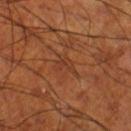The lesion was photographed on a routine skin check and not biopsied; there is no pathology result. Located on the right thigh. A region of skin cropped from a whole-body photographic capture, roughly 15 mm wide. The tile uses cross-polarized illumination. A male patient aged 68–72.